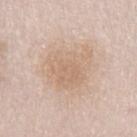Q: What are the patient's age and sex?
A: male, aged approximately 65
Q: How was this image acquired?
A: total-body-photography crop, ~15 mm field of view
Q: Lesion location?
A: the arm
Q: What did automated image analysis measure?
A: a footprint of about 22 mm², a shape eccentricity near 0.7, and a symmetry-axis asymmetry near 0.3; a border-irregularity index near 4/10, a color-variation rating of about 2.5/10, and peripheral color asymmetry of about 1